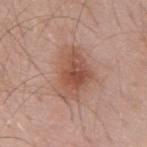Assessment:
The lesion was tiled from a total-body skin photograph and was not biopsied.
Image and clinical context:
Approximately 6 mm at its widest. Imaged with white-light lighting. The subject is a male aged 53 to 57. Cropped from a total-body skin-imaging series; the visible field is about 15 mm. On the mid back. Automated image analysis of the tile measured a lesion area of about 18 mm², an eccentricity of roughly 0.75, and a symmetry-axis asymmetry near 0.3. And it measured a mean CIELAB color near L≈53 a*≈22 b*≈28 and a normalized lesion–skin contrast near 7.5. It also reported an automated nevus-likeness rating near 90 out of 100 and a lesion-detection confidence of about 100/100.